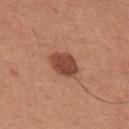Q: Was this lesion biopsied?
A: catalogued during a skin exam; not biopsied
Q: What is the anatomic site?
A: the front of the torso
Q: How was this image acquired?
A: 15 mm crop, total-body photography
Q: Illumination type?
A: white-light illumination
Q: Who is the patient?
A: male, aged around 35